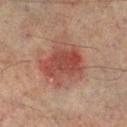Cropped from a total-body skin-imaging series; the visible field is about 15 mm. The lesion's longest dimension is about 5.5 mm. A male subject, about 75 years old. Imaged with cross-polarized lighting. The lesion is located on the left lower leg.